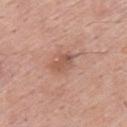| key | value |
|---|---|
| workup | total-body-photography surveillance lesion; no biopsy |
| image-analysis metrics | a symmetry-axis asymmetry near 0.25; a within-lesion color-variation index near 3.5/10 and a peripheral color-asymmetry measure near 1.5 |
| illumination | white-light illumination |
| subject | male, roughly 55 years of age |
| image | 15 mm crop, total-body photography |
| size | about 3 mm |
| body site | the upper back |